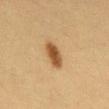| key | value |
|---|---|
| biopsy status | total-body-photography surveillance lesion; no biopsy |
| image | 15 mm crop, total-body photography |
| diameter | ≈3.5 mm |
| lighting | cross-polarized |
| automated lesion analysis | a lesion area of about 6.5 mm², an outline eccentricity of about 0.8 (0 = round, 1 = elongated), and a shape-asymmetry score of about 0.15 (0 = symmetric) |
| patient | female, in their 40s |
| site | the mid back |